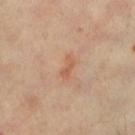Part of a total-body skin-imaging series; this lesion was reviewed on a skin check and was not flagged for biopsy.
The lesion is located on the left lower leg.
A 15 mm close-up extracted from a 3D total-body photography capture.
Longest diameter approximately 2.5 mm.
An algorithmic analysis of the crop reported an eccentricity of roughly 0.9 and a symmetry-axis asymmetry near 0.35. The analysis additionally found a border-irregularity rating of about 3.5/10, a within-lesion color-variation index near 0/10, and a peripheral color-asymmetry measure near 0.
Captured under cross-polarized illumination.
A patient roughly 60 years of age.